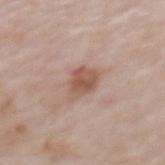notes: imaged on a skin check; not biopsied
body site: the upper back
lesion diameter: ≈3.5 mm
patient: female, approximately 65 years of age
image source: 15 mm crop, total-body photography